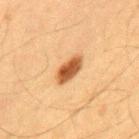This lesion was catalogued during total-body skin photography and was not selected for biopsy.
Imaged with cross-polarized lighting.
A male subject about 65 years old.
A region of skin cropped from a whole-body photographic capture, roughly 15 mm wide.
From the mid back.
Measured at roughly 4 mm in maximum diameter.
An algorithmic analysis of the crop reported border irregularity of about 2 on a 0–10 scale, internal color variation of about 3 on a 0–10 scale, and peripheral color asymmetry of about 1. It also reported a lesion-detection confidence of about 100/100.